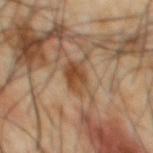Q: Was this lesion biopsied?
A: catalogued during a skin exam; not biopsied
Q: Lesion size?
A: about 3.5 mm
Q: What lighting was used for the tile?
A: cross-polarized illumination
Q: What are the patient's age and sex?
A: male, aged 63 to 67
Q: What kind of image is this?
A: ~15 mm tile from a whole-body skin photo
Q: What did automated image analysis measure?
A: a footprint of about 7 mm², an outline eccentricity of about 0.75 (0 = round, 1 = elongated), and a symmetry-axis asymmetry near 0.2; an average lesion color of about L≈44 a*≈19 b*≈32 (CIELAB) and roughly 10 lightness units darker than nearby skin; a border-irregularity index near 2.5/10, a color-variation rating of about 5/10, and a peripheral color-asymmetry measure near 2; a nevus-likeness score of about 10/100 and a detector confidence of about 100 out of 100 that the crop contains a lesion
Q: Lesion location?
A: the front of the torso